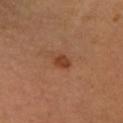biopsy_status: not biopsied; imaged during a skin examination
lesion_size:
  long_diameter_mm_approx: 2.5
patient:
  sex: male
  age_approx: 60
lighting: cross-polarized
image:
  source: total-body photography crop
  field_of_view_mm: 15
automated_metrics:
  cielab_L: 41
  cielab_a: 23
  cielab_b: 33
  vs_skin_darker_L: 9.0
  vs_skin_contrast_norm: 7.5
  nevus_likeness_0_100: 85
  lesion_detection_confidence_0_100: 100
site: head or neck